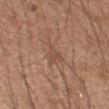biopsy status = total-body-photography surveillance lesion; no biopsy
patient = male, aged approximately 50
lesion size = about 2.5 mm
location = the right forearm
image source = ~15 mm tile from a whole-body skin photo